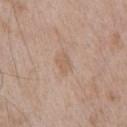No biopsy was performed on this lesion — it was imaged during a full skin examination and was not determined to be concerning. A male patient in their 50s. A 15 mm close-up tile from a total-body photography series done for melanoma screening. From the chest. Automated tile analysis of the lesion measured a lesion area of about 4 mm² and two-axis asymmetry of about 0.15. The analysis additionally found an average lesion color of about L≈60 a*≈16 b*≈29 (CIELAB) and a normalized border contrast of about 5. The software also gave an automated nevus-likeness rating near 0 out of 100. Longest diameter approximately 2.5 mm.